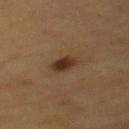• workup · total-body-photography surveillance lesion; no biopsy
• illumination · cross-polarized illumination
• image · total-body-photography crop, ~15 mm field of view
• patient · male, approximately 60 years of age
• site · the mid back